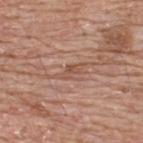Impression: The lesion was photographed on a routine skin check and not biopsied; there is no pathology result. Acquisition and patient details: A lesion tile, about 15 mm wide, cut from a 3D total-body photograph. A male subject approximately 65 years of age. Approximately 3.5 mm at its widest. From the back. Automated tile analysis of the lesion measured two-axis asymmetry of about 0.3. The software also gave an average lesion color of about L≈53 a*≈21 b*≈29 (CIELAB), about 8 CIELAB-L* units darker than the surrounding skin, and a lesion-to-skin contrast of about 6 (normalized; higher = more distinct). And it measured a border-irregularity index near 4/10, internal color variation of about 2 on a 0–10 scale, and peripheral color asymmetry of about 0.5. The analysis additionally found a nevus-likeness score of about 0/100 and a detector confidence of about 75 out of 100 that the crop contains a lesion.